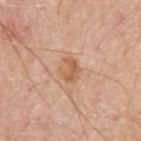patient:
  sex: male
  age_approx: 80
lighting: white-light
automated_metrics:
  vs_skin_darker_L: 9.0
  vs_skin_contrast_norm: 7.0
  color_variation_0_10: 4.0
site: upper back
image:
  source: total-body photography crop
  field_of_view_mm: 15